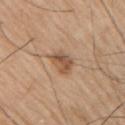The lesion was photographed on a routine skin check and not biopsied; there is no pathology result. From the arm. The recorded lesion diameter is about 4 mm. A male patient, aged 73–77. This image is a 15 mm lesion crop taken from a total-body photograph.